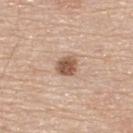Clinical impression:
The lesion was photographed on a routine skin check and not biopsied; there is no pathology result.
Acquisition and patient details:
From the upper back. A male subject, in their 70s. A 15 mm crop from a total-body photograph taken for skin-cancer surveillance.